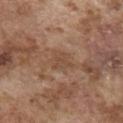Captured during whole-body skin photography for melanoma surveillance; the lesion was not biopsied. On the chest. Automated tile analysis of the lesion measured a lesion area of about 5.5 mm², an outline eccentricity of about 0.55 (0 = round, 1 = elongated), and a shape-asymmetry score of about 0.35 (0 = symmetric). The analysis additionally found a mean CIELAB color near L≈48 a*≈18 b*≈29 and about 6 CIELAB-L* units darker than the surrounding skin. The subject is a male aged approximately 75. A roughly 15 mm field-of-view crop from a total-body skin photograph. This is a white-light tile.